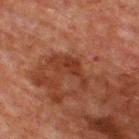Impression: No biopsy was performed on this lesion — it was imaged during a full skin examination and was not determined to be concerning. Clinical summary: Automated image analysis of the tile measured a mean CIELAB color near L≈28 a*≈21 b*≈25, roughly 7 lightness units darker than nearby skin, and a normalized border contrast of about 7. A 15 mm crop from a total-body photograph taken for skin-cancer surveillance. The lesion is on the upper back. The tile uses cross-polarized illumination. A male subject, approximately 60 years of age.